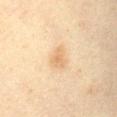Q: Was this lesion biopsied?
A: no biopsy performed (imaged during a skin exam)
Q: What are the patient's age and sex?
A: female, in their mid- to late 50s
Q: How was this image acquired?
A: 15 mm crop, total-body photography
Q: Where on the body is the lesion?
A: the abdomen
Q: Automated lesion metrics?
A: an average lesion color of about L≈61 a*≈15 b*≈34 (CIELAB) and a lesion–skin lightness drop of about 7; a border-irregularity rating of about 3/10, internal color variation of about 1.5 on a 0–10 scale, and a peripheral color-asymmetry measure near 0.5; an automated nevus-likeness rating near 55 out of 100 and a lesion-detection confidence of about 100/100